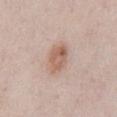| key | value |
|---|---|
| automated lesion analysis | lesion-presence confidence of about 100/100 |
| size | ~4 mm (longest diameter) |
| subject | male, about 60 years old |
| body site | the abdomen |
| imaging modality | 15 mm crop, total-body photography |